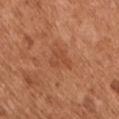Q: Is there a histopathology result?
A: total-body-photography surveillance lesion; no biopsy
Q: What lighting was used for the tile?
A: white-light illumination
Q: Who is the patient?
A: male, in their mid-50s
Q: What did automated image analysis measure?
A: a lesion area of about 5.5 mm², a shape eccentricity near 0.7, and a symmetry-axis asymmetry near 0.45; an average lesion color of about L≈49 a*≈26 b*≈36 (CIELAB) and a lesion–skin lightness drop of about 6; a border-irregularity index near 4.5/10, internal color variation of about 2 on a 0–10 scale, and a peripheral color-asymmetry measure near 0.5; a classifier nevus-likeness of about 0/100
Q: How was this image acquired?
A: total-body-photography crop, ~15 mm field of view
Q: Lesion location?
A: the chest
Q: What is the lesion's diameter?
A: ~3.5 mm (longest diameter)